A region of skin cropped from a whole-body photographic capture, roughly 15 mm wide; the subject is a male in their 60s; the lesion is located on the right forearm — 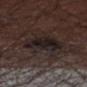{
  "automated_metrics": {
    "area_mm2_approx": 14.0,
    "eccentricity": 0.55,
    "shape_asymmetry": 0.3,
    "nevus_likeness_0_100": 25
  },
  "lesion_size": {
    "long_diameter_mm_approx": 4.5
  },
  "diagnosis": {
    "histopathology": "seborrheic keratosis",
    "malignancy": "benign",
    "taxonomic_path": [
      "Benign",
      "Benign epidermal proliferations",
      "Seborrheic keratosis"
    ]
  }
}